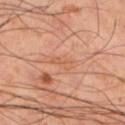Q: Is there a histopathology result?
A: no biopsy performed (imaged during a skin exam)
Q: What lighting was used for the tile?
A: cross-polarized illumination
Q: What is the imaging modality?
A: 15 mm crop, total-body photography
Q: Automated lesion metrics?
A: a shape eccentricity near 0.9 and a shape-asymmetry score of about 0.35 (0 = symmetric); a border-irregularity rating of about 4/10, a within-lesion color-variation index near 1/10, and a peripheral color-asymmetry measure near 0.5; an automated nevus-likeness rating near 0 out of 100 and lesion-presence confidence of about 95/100
Q: Where on the body is the lesion?
A: the left lower leg
Q: Who is the patient?
A: male, in their 50s